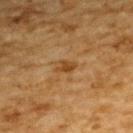No biopsy was performed on this lesion — it was imaged during a full skin examination and was not determined to be concerning. The lesion is located on the upper back. A roughly 15 mm field-of-view crop from a total-body skin photograph. The tile uses cross-polarized illumination. About 2.5 mm across. A male patient aged 83 or older.